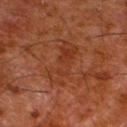Assessment: The lesion was photographed on a routine skin check and not biopsied; there is no pathology result.